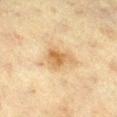Clinical impression: The lesion was photographed on a routine skin check and not biopsied; there is no pathology result. Acquisition and patient details: The total-body-photography lesion software estimated a border-irregularity index near 4.5/10, a within-lesion color-variation index near 4/10, and radial color variation of about 1. It also reported a classifier nevus-likeness of about 60/100. On the right lower leg. A 15 mm close-up tile from a total-body photography series done for melanoma screening. A female patient, in their mid-50s.